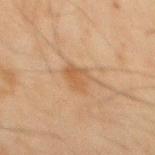Part of a total-body skin-imaging series; this lesion was reviewed on a skin check and was not flagged for biopsy.
The total-body-photography lesion software estimated border irregularity of about 2.5 on a 0–10 scale, internal color variation of about 3 on a 0–10 scale, and radial color variation of about 1. The analysis additionally found a classifier nevus-likeness of about 15/100.
The lesion is on the back.
A 15 mm crop from a total-body photograph taken for skin-cancer surveillance.
The subject is a male approximately 45 years of age.